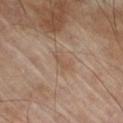body site: the right thigh | acquisition: 15 mm crop, total-body photography | subject: male, approximately 70 years of age.